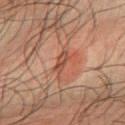The lesion was tiled from a total-body skin photograph and was not biopsied. From the right forearm. A 15 mm close-up extracted from a 3D total-body photography capture. A male patient, aged 48–52.